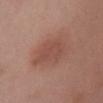follow-up = catalogued during a skin exam; not biopsied
lighting = white-light
site = the chest
lesion size = ~4.5 mm (longest diameter)
image = 15 mm crop, total-body photography
subject = female, in their 40s